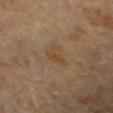biopsy status: no biopsy performed (imaged during a skin exam)
size: ~3 mm (longest diameter)
subject: female, aged 78 to 82
image: 15 mm crop, total-body photography
body site: the right forearm